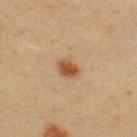Part of a total-body skin-imaging series; this lesion was reviewed on a skin check and was not flagged for biopsy.
Located on the back.
A 15 mm close-up extracted from a 3D total-body photography capture.
Longest diameter approximately 3 mm.
A male subject in their 40s.
The total-body-photography lesion software estimated a detector confidence of about 100 out of 100 that the crop contains a lesion.